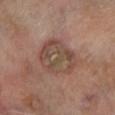Part of a total-body skin-imaging series; this lesion was reviewed on a skin check and was not flagged for biopsy.
Automated tile analysis of the lesion measured an average lesion color of about L≈45 a*≈16 b*≈24 (CIELAB) and roughly 8 lightness units darker than nearby skin. The software also gave lesion-presence confidence of about 100/100.
About 5 mm across.
The tile uses cross-polarized illumination.
A 15 mm close-up tile from a total-body photography series done for melanoma screening.
A male subject roughly 70 years of age.
From the left lower leg.